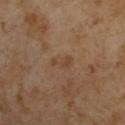| feature | finding |
|---|---|
| workup | catalogued during a skin exam; not biopsied |
| size | ≈3 mm |
| body site | the left upper arm |
| image | total-body-photography crop, ~15 mm field of view |
| subject | male, aged around 55 |
| illumination | cross-polarized |